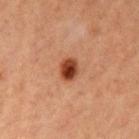Findings:
- workup — catalogued during a skin exam; not biopsied
- size — ~3 mm (longest diameter)
- tile lighting — cross-polarized illumination
- subject — female, about 40 years old
- body site — the left upper arm
- imaging modality — ~15 mm tile from a whole-body skin photo